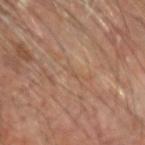Q: Was this lesion biopsied?
A: imaged on a skin check; not biopsied
Q: Lesion location?
A: the head or neck
Q: Patient demographics?
A: male, aged 68 to 72
Q: How was this image acquired?
A: ~15 mm tile from a whole-body skin photo
Q: Automated lesion metrics?
A: a lesion color around L≈52 a*≈18 b*≈30 in CIELAB, roughly 4 lightness units darker than nearby skin, and a normalized border contrast of about 3.5; border irregularity of about 4.5 on a 0–10 scale and peripheral color asymmetry of about 0; an automated nevus-likeness rating near 0 out of 100 and a lesion-detection confidence of about 25/100
Q: How was the tile lit?
A: cross-polarized illumination